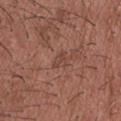Impression:
No biopsy was performed on this lesion — it was imaged during a full skin examination and was not determined to be concerning.
Background:
Measured at roughly 2.5 mm in maximum diameter. A male subject, in their mid- to late 20s. The lesion is on the head or neck. Automated tile analysis of the lesion measured a lesion color around L≈44 a*≈20 b*≈25 in CIELAB. The software also gave border irregularity of about 3.5 on a 0–10 scale, a color-variation rating of about 1/10, and radial color variation of about 0.5. The analysis additionally found an automated nevus-likeness rating near 0 out of 100. Imaged with white-light lighting. A lesion tile, about 15 mm wide, cut from a 3D total-body photograph.